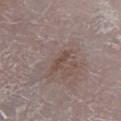{"automated_metrics": {"vs_skin_darker_L": 7.0, "border_irregularity_0_10": 5.5, "color_variation_0_10": 0.0, "peripheral_color_asymmetry": 0.0}, "patient": {"sex": "female", "age_approx": 65}, "image": {"source": "total-body photography crop", "field_of_view_mm": 15}, "site": "right lower leg", "lesion_size": {"long_diameter_mm_approx": 3.0}, "lighting": "white-light"}